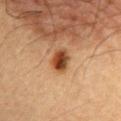| feature | finding |
|---|---|
| follow-up | no biopsy performed (imaged during a skin exam) |
| size | ≈3 mm |
| patient | male, aged 83 or older |
| lighting | cross-polarized |
| image | ~15 mm tile from a whole-body skin photo |
| location | the chest |